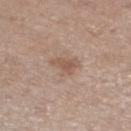Case summary:
– lighting · white-light
– automated lesion analysis · an area of roughly 5.5 mm², an outline eccentricity of about 0.7 (0 = round, 1 = elongated), and a symmetry-axis asymmetry near 0.45; a lesion–skin lightness drop of about 8 and a normalized border contrast of about 6; border irregularity of about 4.5 on a 0–10 scale and a peripheral color-asymmetry measure near 1
– image · total-body-photography crop, ~15 mm field of view
– location · the left lower leg
– subject · male, roughly 70 years of age
– lesion diameter · about 3.5 mm